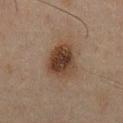Clinical impression:
The lesion was photographed on a routine skin check and not biopsied; there is no pathology result.
Context:
A male subject aged approximately 45. A close-up tile cropped from a whole-body skin photograph, about 15 mm across. The lesion is on the chest. This is a cross-polarized tile. Automated image analysis of the tile measured an area of roughly 13 mm², an outline eccentricity of about 0.65 (0 = round, 1 = elongated), and two-axis asymmetry of about 0.2. It also reported a nevus-likeness score of about 95/100 and lesion-presence confidence of about 100/100.